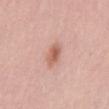{"biopsy_status": "not biopsied; imaged during a skin examination", "lighting": "white-light", "lesion_size": {"long_diameter_mm_approx": 2.5}, "site": "mid back", "patient": {"sex": "male", "age_approx": 55}, "image": {"source": "total-body photography crop", "field_of_view_mm": 15}, "automated_metrics": {"cielab_L": 60, "cielab_a": 24, "cielab_b": 29, "vs_skin_darker_L": 11.0, "vs_skin_contrast_norm": 7.5, "nevus_likeness_0_100": 95, "lesion_detection_confidence_0_100": 100}}